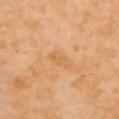A female patient approximately 55 years of age.
Cropped from a whole-body photographic skin survey; the tile spans about 15 mm.
From the chest.